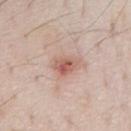Findings:
* workup · no biopsy performed (imaged during a skin exam)
* diameter · ≈3 mm
* imaging modality · ~15 mm crop, total-body skin-cancer survey
* site · the chest
* subject · male, aged 78 to 82
* tile lighting · white-light illumination